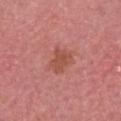Q: Was this lesion biopsied?
A: no biopsy performed (imaged during a skin exam)
Q: Lesion location?
A: the head or neck
Q: Patient demographics?
A: male, aged approximately 55
Q: What kind of image is this?
A: total-body-photography crop, ~15 mm field of view
Q: Lesion size?
A: about 3 mm
Q: Automated lesion metrics?
A: an average lesion color of about L≈51 a*≈28 b*≈29 (CIELAB), a lesion–skin lightness drop of about 8, and a lesion-to-skin contrast of about 6.5 (normalized; higher = more distinct); a border-irregularity index near 3/10 and a peripheral color-asymmetry measure near 0.5